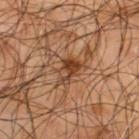Context:
This is a cross-polarized tile. Longest diameter approximately 3.5 mm. A male subject, aged around 65. A 15 mm crop from a total-body photograph taken for skin-cancer surveillance. From the upper back.